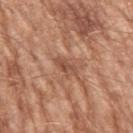follow-up: no biopsy performed (imaged during a skin exam) | patient: male, in their mid-60s | lighting: white-light | automated metrics: an area of roughly 4 mm², a shape eccentricity near 0.85, and two-axis asymmetry of about 0.25; a mean CIELAB color near L≈51 a*≈23 b*≈30, roughly 9 lightness units darker than nearby skin, and a normalized lesion–skin contrast near 6.5; border irregularity of about 2.5 on a 0–10 scale, a color-variation rating of about 5/10, and peripheral color asymmetry of about 2; an automated nevus-likeness rating near 0 out of 100 | lesion size: about 3 mm | anatomic site: the left upper arm | image: ~15 mm crop, total-body skin-cancer survey.